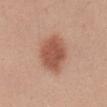Recorded during total-body skin imaging; not selected for excision or biopsy.
Located on the abdomen.
The subject is a female about 40 years old.
Approximately 5.5 mm at its widest.
The lesion-visualizer software estimated a lesion area of about 16 mm², an eccentricity of roughly 0.65, and a symmetry-axis asymmetry near 0.15. The software also gave a mean CIELAB color near L≈54 a*≈24 b*≈30, about 12 CIELAB-L* units darker than the surrounding skin, and a normalized lesion–skin contrast near 8.5. And it measured a within-lesion color-variation index near 3/10 and peripheral color asymmetry of about 1. The software also gave a detector confidence of about 100 out of 100 that the crop contains a lesion.
Cropped from a total-body skin-imaging series; the visible field is about 15 mm.
Imaged with white-light lighting.